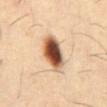Clinical impression: Recorded during total-body skin imaging; not selected for excision or biopsy. Acquisition and patient details: Measured at roughly 5 mm in maximum diameter. Automated tile analysis of the lesion measured a footprint of about 12 mm² and an outline eccentricity of about 0.8 (0 = round, 1 = elongated). And it measured a lesion color around L≈53 a*≈21 b*≈33 in CIELAB, roughly 24 lightness units darker than nearby skin, and a normalized border contrast of about 14.5. The software also gave a within-lesion color-variation index near 9.5/10 and peripheral color asymmetry of about 3. On the abdomen. Imaged with cross-polarized lighting. A close-up tile cropped from a whole-body skin photograph, about 15 mm across. A male patient, aged approximately 55.